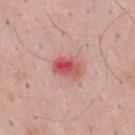Q: Was this lesion biopsied?
A: total-body-photography surveillance lesion; no biopsy
Q: How was the tile lit?
A: white-light illumination
Q: What are the patient's age and sex?
A: male, aged 33–37
Q: What kind of image is this?
A: ~15 mm tile from a whole-body skin photo
Q: Lesion size?
A: ~3.5 mm (longest diameter)
Q: What is the anatomic site?
A: the mid back